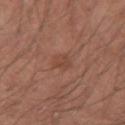Impression:
Part of a total-body skin-imaging series; this lesion was reviewed on a skin check and was not flagged for biopsy.
Context:
About 2.5 mm across. A male patient about 30 years old. From the left forearm. A close-up tile cropped from a whole-body skin photograph, about 15 mm across. Automated image analysis of the tile measured a footprint of about 4 mm², an eccentricity of roughly 0.65, and a symmetry-axis asymmetry near 0.3. And it measured a lesion–skin lightness drop of about 6. It also reported internal color variation of about 2 on a 0–10 scale and radial color variation of about 0.5. And it measured a classifier nevus-likeness of about 5/100. Imaged with white-light lighting.